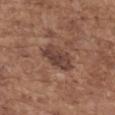{"biopsy_status": "not biopsied; imaged during a skin examination", "lighting": "white-light", "image": {"source": "total-body photography crop", "field_of_view_mm": 15}, "lesion_size": {"long_diameter_mm_approx": 4.5}, "site": "mid back", "patient": {"sex": "female", "age_approx": 65}, "automated_metrics": {"border_irregularity_0_10": 2.5, "color_variation_0_10": 3.0, "peripheral_color_asymmetry": 1.0, "nevus_likeness_0_100": 5, "lesion_detection_confidence_0_100": 100}}